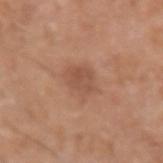biopsy status — imaged on a skin check; not biopsied
patient — male, about 65 years old
lesion size — ≈3.5 mm
anatomic site — the left upper arm
automated metrics — a lesion color around L≈52 a*≈22 b*≈30 in CIELAB and a lesion-to-skin contrast of about 5.5 (normalized; higher = more distinct); a border-irregularity rating of about 2.5/10 and a within-lesion color-variation index near 2.5/10
illumination — white-light
image — 15 mm crop, total-body photography